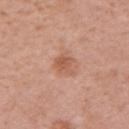• biopsy status · no biopsy performed (imaged during a skin exam)
• location · the right upper arm
• patient · female, aged around 45
• imaging modality · total-body-photography crop, ~15 mm field of view
• automated lesion analysis · an average lesion color of about L≈57 a*≈24 b*≈32 (CIELAB) and a lesion–skin lightness drop of about 9; a border-irregularity index near 3/10, internal color variation of about 2.5 on a 0–10 scale, and peripheral color asymmetry of about 1; an automated nevus-likeness rating near 25 out of 100